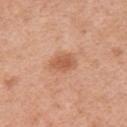Case summary:
* notes: imaged on a skin check; not biopsied
* subject: female, in their 50s
* site: the right upper arm
* acquisition: ~15 mm tile from a whole-body skin photo